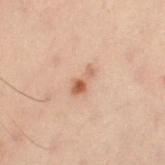notes: catalogued during a skin exam; not biopsied
imaging modality: ~15 mm crop, total-body skin-cancer survey
automated lesion analysis: an eccentricity of roughly 0.9 and a shape-asymmetry score of about 0.4 (0 = symmetric); a lesion color around L≈50 a*≈18 b*≈28 in CIELAB and about 9 CIELAB-L* units darker than the surrounding skin; a border-irregularity rating of about 4/10, a within-lesion color-variation index near 2.5/10, and radial color variation of about 0.5
lesion diameter: ≈3 mm
site: the left thigh
patient: male, aged 48–52
illumination: cross-polarized